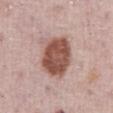A region of skin cropped from a whole-body photographic capture, roughly 15 mm wide.
The tile uses white-light illumination.
A male subject, aged 73 to 77.
The recorded lesion diameter is about 5 mm.
The lesion-visualizer software estimated a lesion area of about 18 mm², a shape eccentricity near 0.5, and two-axis asymmetry of about 0.15. It also reported a mean CIELAB color near L≈51 a*≈22 b*≈25, roughly 16 lightness units darker than nearby skin, and a lesion-to-skin contrast of about 11 (normalized; higher = more distinct).
Located on the abdomen.